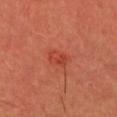Captured during whole-body skin photography for melanoma surveillance; the lesion was not biopsied. Cropped from a whole-body photographic skin survey; the tile spans about 15 mm. Located on the head or neck. The subject is a male approximately 40 years of age. Imaged with cross-polarized lighting. About 2.5 mm across.